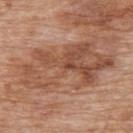Clinical impression:
The lesion was tiled from a total-body skin photograph and was not biopsied.
Image and clinical context:
A roughly 15 mm field-of-view crop from a total-body skin photograph. The total-body-photography lesion software estimated a lesion color around L≈51 a*≈22 b*≈31 in CIELAB and roughly 10 lightness units darker than nearby skin. It also reported a border-irregularity index near 7.5/10, a color-variation rating of about 7/10, and a peripheral color-asymmetry measure near 3. A male subject, aged 78 to 82. On the upper back. This is a white-light tile.